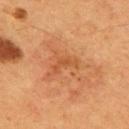{"biopsy_status": "not biopsied; imaged during a skin examination", "patient": {"sex": "male", "age_approx": 55}, "image": {"source": "total-body photography crop", "field_of_view_mm": 15}, "automated_metrics": {"area_mm2_approx": 3.0, "shape_asymmetry": 0.65, "cielab_L": 49, "cielab_a": 26, "cielab_b": 39, "vs_skin_darker_L": 7.0, "vs_skin_contrast_norm": 5.0}, "site": "upper back", "lighting": "cross-polarized"}